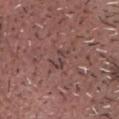Impression: No biopsy was performed on this lesion — it was imaged during a full skin examination and was not determined to be concerning. Context: The total-body-photography lesion software estimated a footprint of about 4 mm², an outline eccentricity of about 0.85 (0 = round, 1 = elongated), and a symmetry-axis asymmetry near 0.35. And it measured a detector confidence of about 85 out of 100 that the crop contains a lesion. A male subject roughly 65 years of age. The lesion is located on the head or neck. Measured at roughly 3 mm in maximum diameter. Captured under white-light illumination. A close-up tile cropped from a whole-body skin photograph, about 15 mm across.